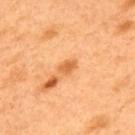Case summary:
- body site: the upper back
- tile lighting: cross-polarized
- diameter: about 2.5 mm
- subject: male, aged 48–52
- image source: ~15 mm crop, total-body skin-cancer survey